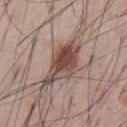Imaged during a routine full-body skin examination; the lesion was not biopsied and no histopathology is available. Approximately 7 mm at its widest. Captured under white-light illumination. The subject is a male aged around 55. A close-up tile cropped from a whole-body skin photograph, about 15 mm across. On the abdomen.